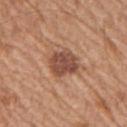An algorithmic analysis of the crop reported a footprint of about 10 mm² and an eccentricity of roughly 0.6. And it measured border irregularity of about 1.5 on a 0–10 scale, a color-variation rating of about 3.5/10, and radial color variation of about 1.5. The software also gave a nevus-likeness score of about 35/100.
The tile uses white-light illumination.
Located on the right upper arm.
Measured at roughly 4 mm in maximum diameter.
The subject is a male aged 63–67.
A region of skin cropped from a whole-body photographic capture, roughly 15 mm wide.